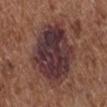| key | value |
|---|---|
| notes | catalogued during a skin exam; not biopsied |